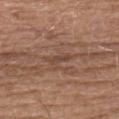Findings:
- follow-up: total-body-photography surveillance lesion; no biopsy
- anatomic site: the upper back
- image source: ~15 mm crop, total-body skin-cancer survey
- subject: male, roughly 75 years of age
- tile lighting: white-light
- diameter: about 2.5 mm